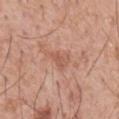notes — total-body-photography surveillance lesion; no biopsy | size — ≈3.5 mm | illumination — white-light | anatomic site — the mid back | image — ~15 mm crop, total-body skin-cancer survey | patient — male, approximately 60 years of age.